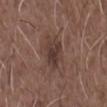Recorded during total-body skin imaging; not selected for excision or biopsy. Approximately 3.5 mm at its widest. A male patient, aged around 50. On the front of the torso. A 15 mm close-up tile from a total-body photography series done for melanoma screening.